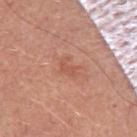• notes — no biopsy performed (imaged during a skin exam)
• image source — ~15 mm crop, total-body skin-cancer survey
• image-analysis metrics — an area of roughly 3 mm², an outline eccentricity of about 0.8 (0 = round, 1 = elongated), and a shape-asymmetry score of about 0.45 (0 = symmetric); a lesion color around L≈55 a*≈26 b*≈31 in CIELAB; internal color variation of about 0.5 on a 0–10 scale and radial color variation of about 0; an automated nevus-likeness rating near 0 out of 100 and a detector confidence of about 100 out of 100 that the crop contains a lesion
• subject — male, aged 53 to 57
• size — ~2.5 mm (longest diameter)
• location — the left upper arm
• lighting — white-light